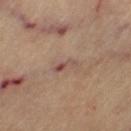notes: total-body-photography surveillance lesion; no biopsy | automated lesion analysis: a classifier nevus-likeness of about 0/100 and a lesion-detection confidence of about 100/100 | image source: total-body-photography crop, ~15 mm field of view | diameter: about 3 mm | patient: female, about 65 years old | tile lighting: cross-polarized | body site: the leg.